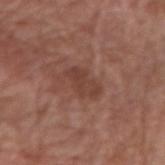| key | value |
|---|---|
| follow-up | total-body-photography surveillance lesion; no biopsy |
| lighting | white-light |
| acquisition | 15 mm crop, total-body photography |
| patient | female, aged around 50 |
| anatomic site | the right forearm |
| size | about 3.5 mm |